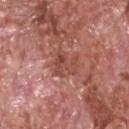Assessment:
This lesion was catalogued during total-body skin photography and was not selected for biopsy.
Context:
Automated tile analysis of the lesion measured an outline eccentricity of about 0.7 (0 = round, 1 = elongated) and a shape-asymmetry score of about 0.55 (0 = symmetric). It also reported an automated nevus-likeness rating near 0 out of 100 and lesion-presence confidence of about 95/100. The lesion is located on the head or neck. The patient is a male roughly 60 years of age. This is a white-light tile. A lesion tile, about 15 mm wide, cut from a 3D total-body photograph.